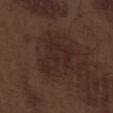Impression: Captured during whole-body skin photography for melanoma surveillance; the lesion was not biopsied.